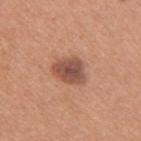An algorithmic analysis of the crop reported a lesion color around L≈51 a*≈23 b*≈28 in CIELAB, roughly 13 lightness units darker than nearby skin, and a normalized lesion–skin contrast near 9.5. The software also gave a classifier nevus-likeness of about 65/100 and lesion-presence confidence of about 100/100. From the right upper arm. Cropped from a whole-body photographic skin survey; the tile spans about 15 mm. The patient is a male aged approximately 30.